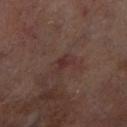Notes:
* follow-up — imaged on a skin check; not biopsied
* image — ~15 mm crop, total-body skin-cancer survey
* automated metrics — a shape eccentricity near 0.8 and a symmetry-axis asymmetry near 0.35; about 5 CIELAB-L* units darker than the surrounding skin and a lesion-to-skin contrast of about 5.5 (normalized; higher = more distinct)
* anatomic site — the right lower leg
* patient — male, aged around 70
* lighting — cross-polarized illumination